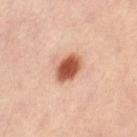{
  "biopsy_status": "not biopsied; imaged during a skin examination",
  "lesion_size": {
    "long_diameter_mm_approx": 3.5
  },
  "lighting": "cross-polarized",
  "site": "right leg",
  "image": {
    "source": "total-body photography crop",
    "field_of_view_mm": 15
  },
  "patient": {
    "sex": "female",
    "age_approx": 40
  }
}